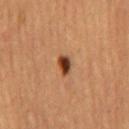No biopsy was performed on this lesion — it was imaged during a full skin examination and was not determined to be concerning.
Automated image analysis of the tile measured an area of roughly 4 mm², an outline eccentricity of about 0.7 (0 = round, 1 = elongated), and a shape-asymmetry score of about 0.3 (0 = symmetric).
The lesion is on the abdomen.
The lesion's longest dimension is about 2.5 mm.
A 15 mm close-up extracted from a 3D total-body photography capture.
The patient is a male aged around 65.